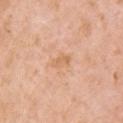Findings:
- lesion diameter: ≈2.5 mm
- acquisition: total-body-photography crop, ~15 mm field of view
- subject: female, aged approximately 70
- tile lighting: white-light
- body site: the right upper arm